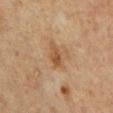workup=imaged on a skin check; not biopsied | site=the mid back | automated lesion analysis=an automated nevus-likeness rating near 10 out of 100 and a lesion-detection confidence of about 100/100 | subject=male, in their 60s | diameter=≈3 mm | tile lighting=cross-polarized illumination | image=~15 mm crop, total-body skin-cancer survey.